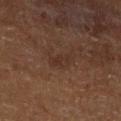The lesion was photographed on a routine skin check and not biopsied; there is no pathology result. Longest diameter approximately 3 mm. A male patient in their mid-70s. This is a cross-polarized tile. A roughly 15 mm field-of-view crop from a total-body skin photograph. Located on the leg.